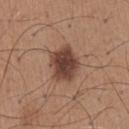Impression: Imaged during a routine full-body skin examination; the lesion was not biopsied and no histopathology is available. Acquisition and patient details: A 15 mm close-up extracted from a 3D total-body photography capture. About 4.5 mm across. The subject is a male in their mid-60s. Captured under white-light illumination. On the chest.